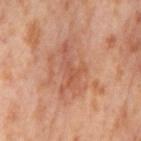Clinical impression:
The lesion was photographed on a routine skin check and not biopsied; there is no pathology result.
Image and clinical context:
The lesion is located on the left thigh. Cropped from a total-body skin-imaging series; the visible field is about 15 mm. The recorded lesion diameter is about 5.5 mm. A female patient aged 53–57. The lesion-visualizer software estimated about 8 CIELAB-L* units darker than the surrounding skin and a normalized border contrast of about 5.5. The analysis additionally found a border-irregularity rating of about 9/10. This is a cross-polarized tile.